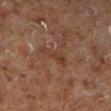Impression:
This lesion was catalogued during total-body skin photography and was not selected for biopsy.